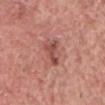This lesion was catalogued during total-body skin photography and was not selected for biopsy.
The lesion is on the chest.
The lesion-visualizer software estimated an average lesion color of about L≈49 a*≈27 b*≈26 (CIELAB) and a normalized border contrast of about 7.5. And it measured border irregularity of about 6.5 on a 0–10 scale, a within-lesion color-variation index near 2/10, and a peripheral color-asymmetry measure near 0.5. The software also gave a classifier nevus-likeness of about 20/100.
The subject is a male aged 58 to 62.
A 15 mm close-up extracted from a 3D total-body photography capture.
The recorded lesion diameter is about 3.5 mm.